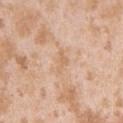The lesion was photographed on a routine skin check and not biopsied; there is no pathology result. Cropped from a whole-body photographic skin survey; the tile spans about 15 mm. Longest diameter approximately 3 mm. The tile uses white-light illumination. An algorithmic analysis of the crop reported a lesion color around L≈66 a*≈19 b*≈35 in CIELAB, about 6 CIELAB-L* units darker than the surrounding skin, and a lesion-to-skin contrast of about 5 (normalized; higher = more distinct). The lesion is located on the left upper arm. A female subject, aged approximately 25.